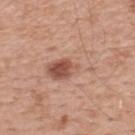biopsy_status: not biopsied; imaged during a skin examination
site: back
lighting: white-light
automated_metrics:
  border_irregularity_0_10: 5.0
  color_variation_0_10: 10.0
  peripheral_color_asymmetry: 5.0
  nevus_likeness_0_100: 90
  lesion_detection_confidence_0_100: 100
patient:
  sex: male
  age_approx: 55
image:
  source: total-body photography crop
  field_of_view_mm: 15